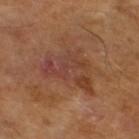Assessment: This lesion was catalogued during total-body skin photography and was not selected for biopsy. Context: The patient is a male in their mid-60s. About 6.5 mm across. A lesion tile, about 15 mm wide, cut from a 3D total-body photograph. Captured under cross-polarized illumination.